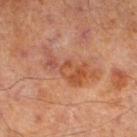- biopsy status · no biopsy performed (imaged during a skin exam)
- illumination · cross-polarized illumination
- anatomic site · the left lower leg
- imaging modality · total-body-photography crop, ~15 mm field of view
- patient · male, aged 68–72